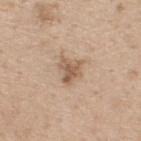Captured during whole-body skin photography for melanoma surveillance; the lesion was not biopsied.
A male subject aged 58–62.
A 15 mm crop from a total-body photograph taken for skin-cancer surveillance.
The lesion is on the upper back.